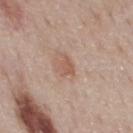Q: Is there a histopathology result?
A: no biopsy performed (imaged during a skin exam)
Q: Where on the body is the lesion?
A: the chest
Q: Illumination type?
A: white-light
Q: Patient demographics?
A: male, in their mid-70s
Q: What is the lesion's diameter?
A: ≈2.5 mm
Q: What kind of image is this?
A: ~15 mm crop, total-body skin-cancer survey